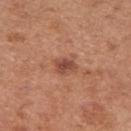Q: Was a biopsy performed?
A: imaged on a skin check; not biopsied
Q: How was the tile lit?
A: white-light
Q: What are the patient's age and sex?
A: female, aged 28 to 32
Q: What is the anatomic site?
A: the arm
Q: Automated lesion metrics?
A: a footprint of about 5.5 mm², an outline eccentricity of about 0.6 (0 = round, 1 = elongated), and a shape-asymmetry score of about 0.25 (0 = symmetric); lesion-presence confidence of about 100/100
Q: What is the lesion's diameter?
A: about 3 mm
Q: How was this image acquired?
A: ~15 mm tile from a whole-body skin photo